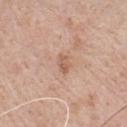Impression:
This lesion was catalogued during total-body skin photography and was not selected for biopsy.
Clinical summary:
The lesion is located on the chest. An algorithmic analysis of the crop reported a lesion color around L≈59 a*≈20 b*≈31 in CIELAB and a lesion–skin lightness drop of about 8. The analysis additionally found a classifier nevus-likeness of about 0/100 and lesion-presence confidence of about 100/100. A male subject, roughly 80 years of age. A lesion tile, about 15 mm wide, cut from a 3D total-body photograph. This is a white-light tile. Approximately 2.5 mm at its widest.